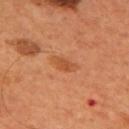biopsy status = catalogued during a skin exam; not biopsied
site = the mid back
subject = male, in their mid- to late 50s
image source = 15 mm crop, total-body photography